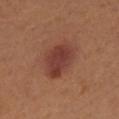Assessment: No biopsy was performed on this lesion — it was imaged during a full skin examination and was not determined to be concerning. Context: The patient is a female aged 53 to 57. The total-body-photography lesion software estimated an area of roughly 15 mm² and an outline eccentricity of about 0.7 (0 = round, 1 = elongated). And it measured a mean CIELAB color near L≈40 a*≈24 b*≈26, about 9 CIELAB-L* units darker than the surrounding skin, and a lesion-to-skin contrast of about 8.5 (normalized; higher = more distinct). The analysis additionally found a nevus-likeness score of about 90/100. Cropped from a total-body skin-imaging series; the visible field is about 15 mm. The lesion is located on the left lower leg.